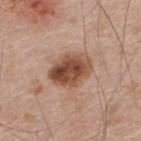Assessment: Part of a total-body skin-imaging series; this lesion was reviewed on a skin check and was not flagged for biopsy. Background: On the upper back. Automated tile analysis of the lesion measured an area of roughly 14 mm² and a shape-asymmetry score of about 0.15 (0 = symmetric). The analysis additionally found a normalized lesion–skin contrast near 11. A male patient about 65 years old. Longest diameter approximately 5 mm. Captured under white-light illumination. A 15 mm close-up tile from a total-body photography series done for melanoma screening.